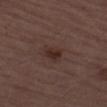{"biopsy_status": "not biopsied; imaged during a skin examination", "patient": {"sex": "male", "age_approx": 70}, "automated_metrics": {"area_mm2_approx": 4.5, "eccentricity": 0.75, "shape_asymmetry": 0.2, "nevus_likeness_0_100": 75}, "lesion_size": {"long_diameter_mm_approx": 3.0}, "lighting": "white-light", "image": {"source": "total-body photography crop", "field_of_view_mm": 15}, "site": "left thigh"}